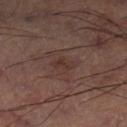<case>
<biopsy_status>not biopsied; imaged during a skin examination</biopsy_status>
<site>left thigh</site>
<image>
  <source>total-body photography crop</source>
  <field_of_view_mm>15</field_of_view_mm>
</image>
</case>